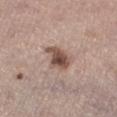The lesion was photographed on a routine skin check and not biopsied; there is no pathology result. On the right lower leg. Captured under white-light illumination. This image is a 15 mm lesion crop taken from a total-body photograph. About 4 mm across. A female subject roughly 65 years of age.